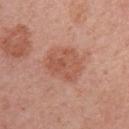The lesion was tiled from a total-body skin photograph and was not biopsied. From the right upper arm. Automated tile analysis of the lesion measured a lesion area of about 16 mm², an eccentricity of roughly 0.55, and a symmetry-axis asymmetry near 0.2. It also reported an average lesion color of about L≈55 a*≈24 b*≈30 (CIELAB), roughly 8 lightness units darker than nearby skin, and a lesion-to-skin contrast of about 6 (normalized; higher = more distinct). The lesion's longest dimension is about 4.5 mm. A female subject, aged around 55. A 15 mm close-up tile from a total-body photography series done for melanoma screening.